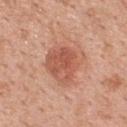Impression:
Recorded during total-body skin imaging; not selected for excision or biopsy.
Context:
A female patient, aged 48 to 52. Cropped from a whole-body photographic skin survey; the tile spans about 15 mm. From the upper back. Measured at roughly 5 mm in maximum diameter.